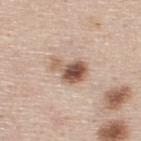notes — imaged on a skin check; not biopsied
subject — male, in their mid- to late 40s
TBP lesion metrics — a mean CIELAB color near L≈56 a*≈18 b*≈28 and a normalized border contrast of about 10; border irregularity of about 4.5 on a 0–10 scale
location — the upper back
image — ~15 mm tile from a whole-body skin photo